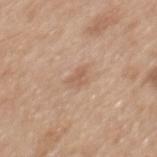Clinical impression: Imaged during a routine full-body skin examination; the lesion was not biopsied and no histopathology is available. Background: Approximately 2.5 mm at its widest. From the back. Captured under white-light illumination. A female subject, aged 38–42. A 15 mm close-up tile from a total-body photography series done for melanoma screening.